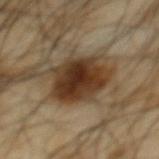notes = total-body-photography surveillance lesion; no biopsy
size = about 5.5 mm
image-analysis metrics = an average lesion color of about L≈32 a*≈15 b*≈28 (CIELAB), a lesion–skin lightness drop of about 15, and a normalized border contrast of about 13; a border-irregularity index near 2/10, a color-variation rating of about 6/10, and radial color variation of about 2
site = the mid back
tile lighting = cross-polarized
image source = 15 mm crop, total-body photography
subject = male, in their mid-60s